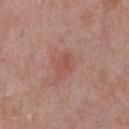Case summary:
– notes: imaged on a skin check; not biopsied
– patient: male, approximately 50 years of age
– size: ~2.5 mm (longest diameter)
– site: the chest
– automated metrics: roughly 6 lightness units darker than nearby skin and a normalized border contrast of about 4.5; internal color variation of about 1.5 on a 0–10 scale and peripheral color asymmetry of about 0.5; a classifier nevus-likeness of about 10/100 and lesion-presence confidence of about 100/100
– image source: ~15 mm crop, total-body skin-cancer survey